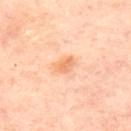<tbp_lesion>
  <biopsy_status>not biopsied; imaged during a skin examination</biopsy_status>
  <image>
    <source>total-body photography crop</source>
    <field_of_view_mm>15</field_of_view_mm>
  </image>
  <site>upper back</site>
  <automated_metrics>
    <area_mm2_approx>3.5</area_mm2_approx>
    <eccentricity>0.75</eccentricity>
    <shape_asymmetry>0.3</shape_asymmetry>
  </automated_metrics>
  <patient>
    <age_approx>55</age_approx>
  </patient>
  <lesion_size>
    <long_diameter_mm_approx>2.5</long_diameter_mm_approx>
  </lesion_size>
</tbp_lesion>